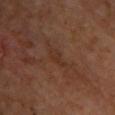The lesion's longest dimension is about 3 mm.
A male subject, roughly 60 years of age.
From the upper back.
A 15 mm close-up tile from a total-body photography series done for melanoma screening.